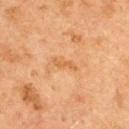follow-up: total-body-photography surveillance lesion; no biopsy | site: the upper back | subject: female, approximately 60 years of age | imaging modality: ~15 mm crop, total-body skin-cancer survey.